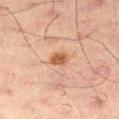Q: Is there a histopathology result?
A: no biopsy performed (imaged during a skin exam)
Q: How large is the lesion?
A: about 2.5 mm
Q: Who is the patient?
A: male, about 50 years old
Q: Lesion location?
A: the right thigh
Q: How was the tile lit?
A: cross-polarized
Q: What is the imaging modality?
A: 15 mm crop, total-body photography